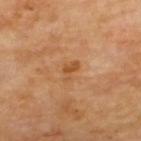  biopsy_status: not biopsied; imaged during a skin examination
  patient:
    sex: male
    age_approx: 70
  lesion_size:
    long_diameter_mm_approx: 2.5
  image:
    source: total-body photography crop
    field_of_view_mm: 15
  site: upper back
  lighting: cross-polarized